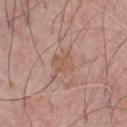Captured during whole-body skin photography for melanoma surveillance; the lesion was not biopsied.
This image is a 15 mm lesion crop taken from a total-body photograph.
The lesion is located on the chest.
A male patient, aged around 65.